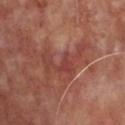Captured during whole-body skin photography for melanoma surveillance; the lesion was not biopsied.
A male subject, about 65 years old.
Measured at roughly 6 mm in maximum diameter.
Captured under white-light illumination.
The total-body-photography lesion software estimated a lesion color around L≈44 a*≈28 b*≈27 in CIELAB, about 7 CIELAB-L* units darker than the surrounding skin, and a normalized border contrast of about 6. And it measured border irregularity of about 10 on a 0–10 scale, a color-variation rating of about 3/10, and peripheral color asymmetry of about 0.5. The software also gave a nevus-likeness score of about 0/100 and lesion-presence confidence of about 90/100.
The lesion is located on the chest.
This image is a 15 mm lesion crop taken from a total-body photograph.